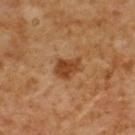workup = catalogued during a skin exam; not biopsied
image source = ~15 mm tile from a whole-body skin photo
subject = male, roughly 60 years of age
location = the back